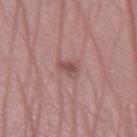  biopsy_status: not biopsied; imaged during a skin examination
  patient:
    sex: male
    age_approx: 60
  image:
    source: total-body photography crop
    field_of_view_mm: 15
  lighting: white-light
  site: left lower leg
  automated_metrics:
    eccentricity: 0.7
    shape_asymmetry: 0.25
    vs_skin_contrast_norm: 6.5
  lesion_size:
    long_diameter_mm_approx: 3.0